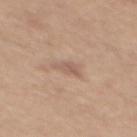{"lighting": "white-light", "site": "mid back", "patient": {"sex": "female", "age_approx": 45}, "image": {"source": "total-body photography crop", "field_of_view_mm": 15}, "lesion_size": {"long_diameter_mm_approx": 3.0}}